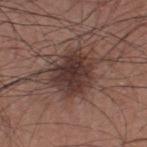Q: Was this lesion biopsied?
A: no biopsy performed (imaged during a skin exam)
Q: Who is the patient?
A: male, aged 33 to 37
Q: Lesion size?
A: ~5.5 mm (longest diameter)
Q: Where on the body is the lesion?
A: the upper back
Q: How was the tile lit?
A: white-light illumination
Q: What did automated image analysis measure?
A: an outline eccentricity of about 0.55 (0 = round, 1 = elongated) and two-axis asymmetry of about 0.2; border irregularity of about 2.5 on a 0–10 scale, a within-lesion color-variation index near 4.5/10, and peripheral color asymmetry of about 1.5; an automated nevus-likeness rating near 80 out of 100
Q: What kind of image is this?
A: ~15 mm tile from a whole-body skin photo